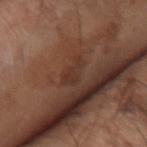A male patient about 70 years old. The lesion is on the arm. About 3 mm across. A roughly 15 mm field-of-view crop from a total-body skin photograph.